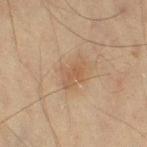Assessment: Captured during whole-body skin photography for melanoma surveillance; the lesion was not biopsied. Clinical summary: Located on the left thigh. Captured under cross-polarized illumination. The lesion-visualizer software estimated a lesion color around L≈49 a*≈16 b*≈29 in CIELAB and roughly 6 lightness units darker than nearby skin. The software also gave a border-irregularity rating of about 3/10, internal color variation of about 2.5 on a 0–10 scale, and radial color variation of about 1. The software also gave lesion-presence confidence of about 100/100. This image is a 15 mm lesion crop taken from a total-body photograph. A female patient roughly 55 years of age.